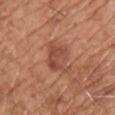{
  "biopsy_status": "not biopsied; imaged during a skin examination",
  "patient": {
    "sex": "male",
    "age_approx": 65
  },
  "lighting": "white-light",
  "site": "front of the torso",
  "image": {
    "source": "total-body photography crop",
    "field_of_view_mm": 15
  }
}